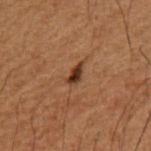Q: Was this lesion biopsied?
A: catalogued during a skin exam; not biopsied
Q: What kind of image is this?
A: ~15 mm crop, total-body skin-cancer survey
Q: Who is the patient?
A: male, aged approximately 50
Q: Lesion location?
A: the left upper arm
Q: Lesion size?
A: about 2.5 mm
Q: Illumination type?
A: cross-polarized